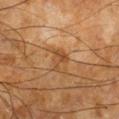<tbp_lesion>
  <biopsy_status>not biopsied; imaged during a skin examination</biopsy_status>
  <site>leg</site>
  <image>
    <source>total-body photography crop</source>
    <field_of_view_mm>15</field_of_view_mm>
  </image>
  <patient>
    <sex>male</sex>
    <age_approx>65</age_approx>
  </patient>
  <lighting>cross-polarized</lighting>
  <lesion_size>
    <long_diameter_mm_approx>2.5</long_diameter_mm_approx>
  </lesion_size>
</tbp_lesion>